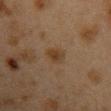| key | value |
|---|---|
| biopsy status | imaged on a skin check; not biopsied |
| diameter | ≈2.5 mm |
| illumination | cross-polarized |
| image source | total-body-photography crop, ~15 mm field of view |
| subject | female, about 40 years old |
| location | the front of the torso |